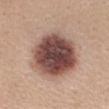{"biopsy_status": "not biopsied; imaged during a skin examination", "site": "mid back", "image": {"source": "total-body photography crop", "field_of_view_mm": 15}, "lighting": "white-light", "lesion_size": {"long_diameter_mm_approx": 7.5}, "patient": {"sex": "female", "age_approx": 35}}